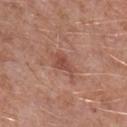Background: This is a white-light tile. The lesion is on the left lower leg. Automated image analysis of the tile measured border irregularity of about 4.5 on a 0–10 scale, a within-lesion color-variation index near 2/10, and peripheral color asymmetry of about 0.5. A close-up tile cropped from a whole-body skin photograph, about 15 mm across. A male patient, aged approximately 60.